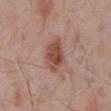Clinical impression:
Imaged during a routine full-body skin examination; the lesion was not biopsied and no histopathology is available.
Clinical summary:
The lesion-visualizer software estimated a border-irregularity index near 2/10, internal color variation of about 4.5 on a 0–10 scale, and peripheral color asymmetry of about 1.5. The software also gave an automated nevus-likeness rating near 85 out of 100 and a detector confidence of about 100 out of 100 that the crop contains a lesion. The lesion is located on the abdomen. Captured under white-light illumination. Longest diameter approximately 4 mm. The patient is a male aged 68–72. Cropped from a total-body skin-imaging series; the visible field is about 15 mm.